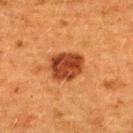{
  "biopsy_status": "not biopsied; imaged during a skin examination",
  "automated_metrics": {
    "border_irregularity_0_10": 1.5,
    "color_variation_0_10": 4.5,
    "peripheral_color_asymmetry": 1.5
  },
  "image": {
    "source": "total-body photography crop",
    "field_of_view_mm": 15
  },
  "lighting": "cross-polarized",
  "patient": {
    "sex": "female",
    "age_approx": 40
  },
  "lesion_size": {
    "long_diameter_mm_approx": 4.0
  },
  "site": "upper back"
}